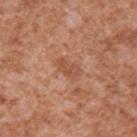| key | value |
|---|---|
| patient | male, aged around 45 |
| imaging modality | total-body-photography crop, ~15 mm field of view |
| image-analysis metrics | a footprint of about 3.5 mm², an outline eccentricity of about 0.85 (0 = round, 1 = elongated), and two-axis asymmetry of about 0.45; an average lesion color of about L≈51 a*≈24 b*≈33 (CIELAB), about 8 CIELAB-L* units darker than the surrounding skin, and a normalized lesion–skin contrast near 5.5 |
| site | the right upper arm |
| lighting | white-light illumination |
| lesion diameter | ~2.5 mm (longest diameter) |